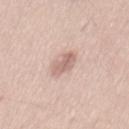biopsy status: total-body-photography surveillance lesion; no biopsy | patient: male, aged 43–47 | anatomic site: the mid back | imaging modality: total-body-photography crop, ~15 mm field of view.